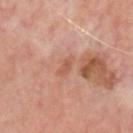Assessment:
No biopsy was performed on this lesion — it was imaged during a full skin examination and was not determined to be concerning.
Clinical summary:
The lesion is located on the head or neck. Cropped from a total-body skin-imaging series; the visible field is about 15 mm. An algorithmic analysis of the crop reported a footprint of about 3 mm², an eccentricity of roughly 0.95, and a symmetry-axis asymmetry near 0.3. The software also gave a nevus-likeness score of about 0/100 and lesion-presence confidence of about 95/100. The patient is a male aged approximately 65.